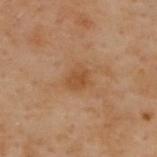No biopsy was performed on this lesion — it was imaged during a full skin examination and was not determined to be concerning. A female patient aged around 60. This is a cross-polarized tile. The lesion's longest dimension is about 3 mm. A 15 mm close-up extracted from a 3D total-body photography capture. The lesion is on the upper back.